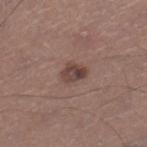Q: Is there a histopathology result?
A: imaged on a skin check; not biopsied
Q: What did automated image analysis measure?
A: an area of roughly 5 mm², a shape eccentricity near 0.65, and a symmetry-axis asymmetry near 0.25; a lesion color around L≈42 a*≈18 b*≈21 in CIELAB, about 10 CIELAB-L* units darker than the surrounding skin, and a normalized border contrast of about 8.5; lesion-presence confidence of about 100/100
Q: Illumination type?
A: white-light
Q: What are the patient's age and sex?
A: male, about 45 years old
Q: Lesion location?
A: the leg
Q: How was this image acquired?
A: ~15 mm tile from a whole-body skin photo
Q: How large is the lesion?
A: ~3 mm (longest diameter)